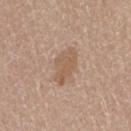Captured during whole-body skin photography for melanoma surveillance; the lesion was not biopsied. About 4 mm across. Located on the leg. A female subject, approximately 70 years of age. Automated image analysis of the tile measured a lesion–skin lightness drop of about 8 and a normalized border contrast of about 6. A close-up tile cropped from a whole-body skin photograph, about 15 mm across. The tile uses white-light illumination.